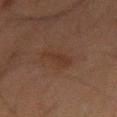follow-up = imaged on a skin check; not biopsied | site = the mid back | patient = male, roughly 65 years of age | illumination = cross-polarized | acquisition = ~15 mm crop, total-body skin-cancer survey.